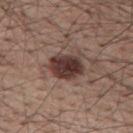Assessment:
This lesion was catalogued during total-body skin photography and was not selected for biopsy.
Clinical summary:
This is a white-light tile. A region of skin cropped from a whole-body photographic capture, roughly 15 mm wide. From the mid back. The lesion-visualizer software estimated a nevus-likeness score of about 90/100 and a lesion-detection confidence of about 100/100. The patient is a male aged 58 to 62. About 4.5 mm across.